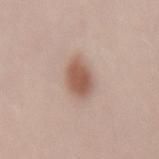notes: total-body-photography surveillance lesion; no biopsy
lesion size: about 4 mm
site: the lower back
patient: male, approximately 25 years of age
imaging modality: ~15 mm tile from a whole-body skin photo